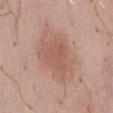Cropped from a whole-body photographic skin survey; the tile spans about 15 mm. On the front of the torso. A male subject in their 40s.